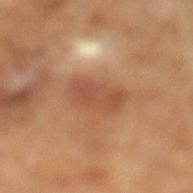{
  "automated_metrics": {
    "cielab_L": 50,
    "cielab_a": 23,
    "cielab_b": 33,
    "peripheral_color_asymmetry": 1.0,
    "nevus_likeness_0_100": 5,
    "lesion_detection_confidence_0_100": 100
  },
  "image": {
    "source": "total-body photography crop",
    "field_of_view_mm": 15
  },
  "lesion_size": {
    "long_diameter_mm_approx": 4.5
  },
  "lighting": "cross-polarized",
  "patient": {
    "sex": "male",
    "age_approx": 65
  }
}